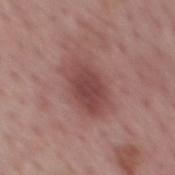The tile uses white-light illumination. Automated image analysis of the tile measured a border-irregularity index near 2/10, internal color variation of about 3 on a 0–10 scale, and peripheral color asymmetry of about 1. The subject is a male in their mid- to late 70s. The lesion is located on the mid back. This image is a 15 mm lesion crop taken from a total-body photograph. The recorded lesion diameter is about 6 mm.